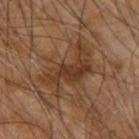Part of a total-body skin-imaging series; this lesion was reviewed on a skin check and was not flagged for biopsy. A 15 mm close-up extracted from a 3D total-body photography capture. The lesion is on the right upper arm. The tile uses cross-polarized illumination. Approximately 7 mm at its widest. A male subject aged approximately 65.